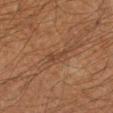The lesion was tiled from a total-body skin photograph and was not biopsied.
A male subject aged 48 to 52.
The lesion is located on the arm.
A close-up tile cropped from a whole-body skin photograph, about 15 mm across.